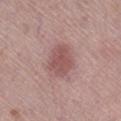Clinical impression: This lesion was catalogued during total-body skin photography and was not selected for biopsy. Acquisition and patient details: The lesion's longest dimension is about 4 mm. A 15 mm close-up extracted from a 3D total-body photography capture. The tile uses white-light illumination. The lesion is located on the right lower leg. The patient is a female aged 63–67.